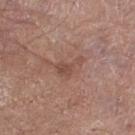Recorded during total-body skin imaging; not selected for excision or biopsy. Located on the right thigh. A 15 mm close-up tile from a total-body photography series done for melanoma screening. A male subject, aged approximately 75.